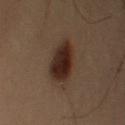follow-up: total-body-photography surveillance lesion; no biopsy
subject: male, about 40 years old
image: total-body-photography crop, ~15 mm field of view
lighting: cross-polarized illumination
diameter: ≈4.5 mm
body site: the left upper arm